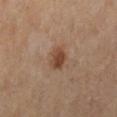Findings:
* biopsy status — total-body-photography surveillance lesion; no biopsy
* lighting — cross-polarized illumination
* imaging modality — total-body-photography crop, ~15 mm field of view
* subject — female, aged approximately 50
* anatomic site — the left thigh
* size — ~3 mm (longest diameter)